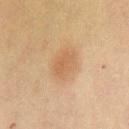Part of a total-body skin-imaging series; this lesion was reviewed on a skin check and was not flagged for biopsy.